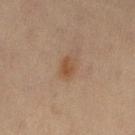No biopsy was performed on this lesion — it was imaged during a full skin examination and was not determined to be concerning.
Located on the left thigh.
Imaged with cross-polarized lighting.
A female subject aged approximately 45.
The recorded lesion diameter is about 2.5 mm.
A roughly 15 mm field-of-view crop from a total-body skin photograph.